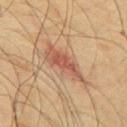{"biopsy_status": "not biopsied; imaged during a skin examination", "site": "right upper arm", "lesion_size": {"long_diameter_mm_approx": 6.0}, "automated_metrics": {"area_mm2_approx": 11.0, "eccentricity": 0.9, "nevus_likeness_0_100": 0, "lesion_detection_confidence_0_100": 100}, "patient": {"sex": "male", "age_approx": 75}, "image": {"source": "total-body photography crop", "field_of_view_mm": 15}, "lighting": "cross-polarized"}